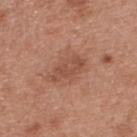Notes:
• follow-up: total-body-photography surveillance lesion; no biopsy
• patient: male, roughly 30 years of age
• diameter: about 4.5 mm
• body site: the upper back
• image source: total-body-photography crop, ~15 mm field of view